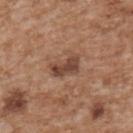The lesion was tiled from a total-body skin photograph and was not biopsied. The tile uses white-light illumination. A roughly 15 mm field-of-view crop from a total-body skin photograph. Located on the upper back. An algorithmic analysis of the crop reported a nevus-likeness score of about 30/100 and lesion-presence confidence of about 100/100. A male patient, about 65 years old. Approximately 3.5 mm at its widest.